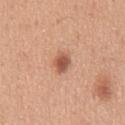This lesion was catalogued during total-body skin photography and was not selected for biopsy.
This image is a 15 mm lesion crop taken from a total-body photograph.
The lesion is on the mid back.
About 2.5 mm across.
A male subject about 50 years old.